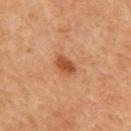<record>
  <image>
    <source>total-body photography crop</source>
    <field_of_view_mm>15</field_of_view_mm>
  </image>
  <site>upper back</site>
  <automated_metrics>
    <area_mm2_approx>4.0</area_mm2_approx>
    <eccentricity>0.8</eccentricity>
    <shape_asymmetry>0.3</shape_asymmetry>
    <cielab_L>48</cielab_L>
    <cielab_a>27</cielab_a>
    <cielab_b>36</cielab_b>
    <vs_skin_darker_L>11.0</vs_skin_darker_L>
    <vs_skin_contrast_norm>8.5</vs_skin_contrast_norm>
    <color_variation_0_10>2.0</color_variation_0_10>
    <lesion_detection_confidence_0_100>100</lesion_detection_confidence_0_100>
  </automated_metrics>
  <patient>
    <sex>male</sex>
    <age_approx>45</age_approx>
  </patient>
</record>